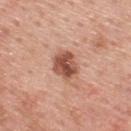Q: Was this lesion biopsied?
A: no biopsy performed (imaged during a skin exam)
Q: Patient demographics?
A: male, in their 60s
Q: What is the imaging modality?
A: ~15 mm crop, total-body skin-cancer survey
Q: What is the lesion's diameter?
A: about 3.5 mm
Q: What lighting was used for the tile?
A: white-light
Q: Lesion location?
A: the upper back
Q: What did automated image analysis measure?
A: an area of roughly 8 mm² and an eccentricity of roughly 0.55; a border-irregularity index near 2/10, a color-variation rating of about 6/10, and peripheral color asymmetry of about 2.5; a classifier nevus-likeness of about 75/100 and a detector confidence of about 100 out of 100 that the crop contains a lesion